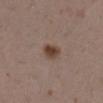Q: Is there a histopathology result?
A: imaged on a skin check; not biopsied
Q: How was this image acquired?
A: 15 mm crop, total-body photography
Q: Where on the body is the lesion?
A: the right lower leg
Q: How was the tile lit?
A: white-light illumination
Q: Patient demographics?
A: female, approximately 30 years of age
Q: Lesion size?
A: ~3 mm (longest diameter)